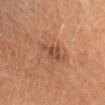Captured during whole-body skin photography for melanoma surveillance; the lesion was not biopsied. Located on the upper back. The lesion-visualizer software estimated two-axis asymmetry of about 0.3. And it measured a lesion color around L≈47 a*≈22 b*≈31 in CIELAB, about 9 CIELAB-L* units darker than the surrounding skin, and a normalized lesion–skin contrast near 7. It also reported a border-irregularity index near 4/10, a color-variation rating of about 2.5/10, and radial color variation of about 1. About 3.5 mm across. A close-up tile cropped from a whole-body skin photograph, about 15 mm across. A female subject, about 50 years old. This is a cross-polarized tile.